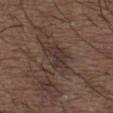A 15 mm crop from a total-body photograph taken for skin-cancer surveillance.
Captured under white-light illumination.
Located on the upper back.
The subject is a male about 50 years old.